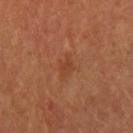{
  "image": {
    "source": "total-body photography crop",
    "field_of_view_mm": 15
  },
  "site": "left arm",
  "patient": {
    "sex": "female",
    "age_approx": 65
  },
  "lesion_size": {
    "long_diameter_mm_approx": 2.5
  }
}